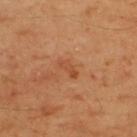<lesion>
<biopsy_status>not biopsied; imaged during a skin examination</biopsy_status>
<lighting>cross-polarized</lighting>
<site>upper back</site>
<lesion_size>
  <long_diameter_mm_approx>2.5</long_diameter_mm_approx>
</lesion_size>
<automated_metrics>
  <eccentricity>0.9</eccentricity>
  <shape_asymmetry>0.45</shape_asymmetry>
  <lesion_detection_confidence_0_100>100</lesion_detection_confidence_0_100>
</automated_metrics>
<patient>
  <sex>male</sex>
  <age_approx>45</age_approx>
</patient>
<image>
  <source>total-body photography crop</source>
  <field_of_view_mm>15</field_of_view_mm>
</image>
</lesion>